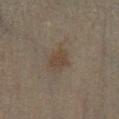No biopsy was performed on this lesion — it was imaged during a full skin examination and was not determined to be concerning. The recorded lesion diameter is about 3 mm. A roughly 15 mm field-of-view crop from a total-body skin photograph. A male subject, approximately 45 years of age. An algorithmic analysis of the crop reported a shape eccentricity near 0.7 and a shape-asymmetry score of about 0.25 (0 = symmetric). And it measured a peripheral color-asymmetry measure near 0.5. Imaged with cross-polarized lighting. The lesion is located on the left lower leg.